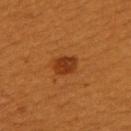Case summary:
- biopsy status · no biopsy performed (imaged during a skin exam)
- diameter · about 2.5 mm
- lighting · cross-polarized illumination
- subject · female, aged around 30
- image source · ~15 mm crop, total-body skin-cancer survey
- site · the back